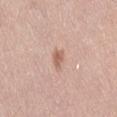This lesion was catalogued during total-body skin photography and was not selected for biopsy.
The tile uses white-light illumination.
Measured at roughly 2.5 mm in maximum diameter.
A 15 mm crop from a total-body photograph taken for skin-cancer surveillance.
The lesion is on the lower back.
A female patient approximately 40 years of age.